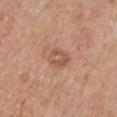<tbp_lesion>
<biopsy_status>not biopsied; imaged during a skin examination</biopsy_status>
<patient>
  <sex>male</sex>
  <age_approx>65</age_approx>
</patient>
<site>right upper arm</site>
<image>
  <source>total-body photography crop</source>
  <field_of_view_mm>15</field_of_view_mm>
</image>
<automated_metrics>
  <shape_asymmetry>0.25</shape_asymmetry>
  <cielab_L>54</cielab_L>
  <cielab_a>21</cielab_a>
  <cielab_b>31</cielab_b>
</automated_metrics>
<lesion_size>
  <long_diameter_mm_approx>2.5</long_diameter_mm_approx>
</lesion_size>
<lighting>white-light</lighting>
</tbp_lesion>